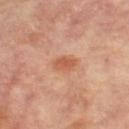Clinical impression:
Recorded during total-body skin imaging; not selected for excision or biopsy.
Background:
This is a cross-polarized tile. The lesion is on the right leg. Approximately 3 mm at its widest. A close-up tile cropped from a whole-body skin photograph, about 15 mm across. A female patient, about 65 years old.